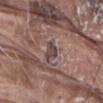illumination: white-light
location: the abdomen
diameter: about 2.5 mm
image: ~15 mm tile from a whole-body skin photo
subject: male, about 80 years old